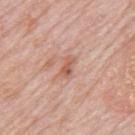- notes: total-body-photography surveillance lesion; no biopsy
- patient: male, aged 78 to 82
- lesion diameter: ~2.5 mm (longest diameter)
- acquisition: ~15 mm tile from a whole-body skin photo
- site: the back